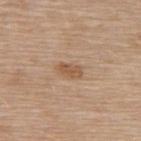biopsy status: no biopsy performed (imaged during a skin exam)